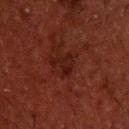{
  "biopsy_status": "not biopsied; imaged during a skin examination",
  "patient": {
    "sex": "male",
    "age_approx": 50
  },
  "image": {
    "source": "total-body photography crop",
    "field_of_view_mm": 15
  },
  "lesion_size": {
    "long_diameter_mm_approx": 3.0
  },
  "site": "upper back",
  "lighting": "cross-polarized"
}